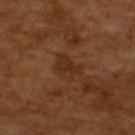{"biopsy_status": "not biopsied; imaged during a skin examination", "image": {"source": "total-body photography crop", "field_of_view_mm": 15}, "patient": {"sex": "male", "age_approx": 65}, "automated_metrics": {"area_mm2_approx": 5.5, "eccentricity": 0.75, "shape_asymmetry": 0.35, "border_irregularity_0_10": 3.5, "color_variation_0_10": 1.5, "peripheral_color_asymmetry": 0.5}, "lighting": "cross-polarized", "lesion_size": {"long_diameter_mm_approx": 3.5}}